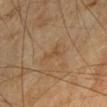Q: Was this lesion biopsied?
A: catalogued during a skin exam; not biopsied
Q: Patient demographics?
A: male, aged approximately 70
Q: How large is the lesion?
A: about 2.5 mm
Q: What is the imaging modality?
A: ~15 mm tile from a whole-body skin photo
Q: Where on the body is the lesion?
A: the arm